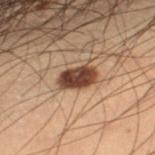patient:
  sex: male
  age_approx: 55
site: right lower leg
lighting: cross-polarized
lesion_size:
  long_diameter_mm_approx: 3.5
automated_metrics:
  eccentricity: 0.75
  shape_asymmetry: 0.15
  color_variation_0_10: 3.5
  peripheral_color_asymmetry: 1.5
  nevus_likeness_0_100: 100
  lesion_detection_confidence_0_100: 100
image:
  source: total-body photography crop
  field_of_view_mm: 15